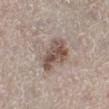Clinical impression:
No biopsy was performed on this lesion — it was imaged during a full skin examination and was not determined to be concerning.
Clinical summary:
Captured under white-light illumination. Automated tile analysis of the lesion measured an eccentricity of roughly 0.85 and a symmetry-axis asymmetry near 0.3. The software also gave internal color variation of about 5 on a 0–10 scale and peripheral color asymmetry of about 2. The software also gave an automated nevus-likeness rating near 50 out of 100. A lesion tile, about 15 mm wide, cut from a 3D total-body photograph. Approximately 5.5 mm at its widest. A female subject aged around 50. From the left lower leg.